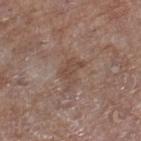Part of a total-body skin-imaging series; this lesion was reviewed on a skin check and was not flagged for biopsy. Longest diameter approximately 3 mm. A 15 mm crop from a total-body photograph taken for skin-cancer surveillance. On the right lower leg. A female patient roughly 75 years of age. This is a white-light tile.